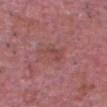notes: catalogued during a skin exam; not biopsied | tile lighting: white-light | image source: ~15 mm tile from a whole-body skin photo | location: the head or neck | patient: male, aged 63 to 67 | lesion diameter: ≈3 mm.